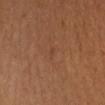Clinical impression: No biopsy was performed on this lesion — it was imaged during a full skin examination and was not determined to be concerning. Image and clinical context: A lesion tile, about 15 mm wide, cut from a 3D total-body photograph. Imaged with cross-polarized lighting. A female patient aged around 35. The recorded lesion diameter is about 1 mm. The total-body-photography lesion software estimated a footprint of about 1 mm², an outline eccentricity of about 0.7 (0 = round, 1 = elongated), and a symmetry-axis asymmetry near 0.35. It also reported a lesion color around L≈40 a*≈21 b*≈30 in CIELAB, about 4 CIELAB-L* units darker than the surrounding skin, and a normalized border contrast of about 3. It also reported a classifier nevus-likeness of about 0/100 and a detector confidence of about 100 out of 100 that the crop contains a lesion. The lesion is on the left forearm.